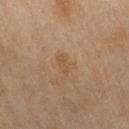The tile uses cross-polarized illumination. The subject is a female about 60 years old. From the right thigh. This image is a 15 mm lesion crop taken from a total-body photograph. The recorded lesion diameter is about 3 mm.